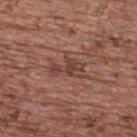– biopsy status · catalogued during a skin exam; not biopsied
– lighting · white-light illumination
– lesion diameter · ≈4.5 mm
– anatomic site · the upper back
– image · ~15 mm crop, total-body skin-cancer survey
– patient · female, in their mid- to late 60s